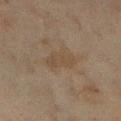<record>
  <biopsy_status>not biopsied; imaged during a skin examination</biopsy_status>
  <site>right lower leg</site>
  <patient>
    <sex>female</sex>
    <age_approx>55</age_approx>
  </patient>
  <image>
    <source>total-body photography crop</source>
    <field_of_view_mm>15</field_of_view_mm>
  </image>
  <automated_metrics>
    <area_mm2_approx>6.0</area_mm2_approx>
    <shape_asymmetry>0.25</shape_asymmetry>
  </automated_metrics>
  <lesion_size>
    <long_diameter_mm_approx>3.5</long_diameter_mm_approx>
  </lesion_size>
</record>